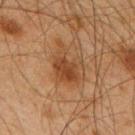| feature | finding |
|---|---|
| notes | catalogued during a skin exam; not biopsied |
| TBP lesion metrics | a shape eccentricity near 0.75 and a shape-asymmetry score of about 0.4 (0 = symmetric); a classifier nevus-likeness of about 40/100 and a detector confidence of about 100 out of 100 that the crop contains a lesion |
| body site | the upper back |
| imaging modality | 15 mm crop, total-body photography |
| subject | male, aged around 65 |
| illumination | cross-polarized illumination |
| lesion size | ~5.5 mm (longest diameter) |